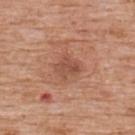Assessment:
The lesion was tiled from a total-body skin photograph and was not biopsied.
Context:
From the upper back. A 15 mm close-up tile from a total-body photography series done for melanoma screening. A male subject, approximately 60 years of age.